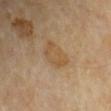Impression: This lesion was catalogued during total-body skin photography and was not selected for biopsy. Context: The lesion-visualizer software estimated a footprint of about 7 mm². The software also gave a lesion-detection confidence of about 100/100. The subject is a male aged around 70. Imaged with cross-polarized lighting. Longest diameter approximately 4 mm. A region of skin cropped from a whole-body photographic capture, roughly 15 mm wide. On the left upper arm.